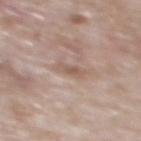Recorded during total-body skin imaging; not selected for excision or biopsy.
The lesion is on the mid back.
This is a white-light tile.
The lesion-visualizer software estimated a shape eccentricity near 0.85 and a symmetry-axis asymmetry near 0.2. It also reported border irregularity of about 3 on a 0–10 scale, a color-variation rating of about 2.5/10, and peripheral color asymmetry of about 1.
A close-up tile cropped from a whole-body skin photograph, about 15 mm across.
The patient is a male aged 58 to 62.